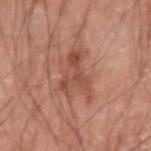The lesion was photographed on a routine skin check and not biopsied; there is no pathology result.
On the right upper arm.
The total-body-photography lesion software estimated a footprint of about 9.5 mm² and an eccentricity of roughly 0.8. The analysis additionally found a border-irregularity rating of about 8/10, internal color variation of about 4 on a 0–10 scale, and radial color variation of about 1. It also reported a classifier nevus-likeness of about 0/100 and a lesion-detection confidence of about 100/100.
A lesion tile, about 15 mm wide, cut from a 3D total-body photograph.
About 5.5 mm across.
Imaged with white-light lighting.
A male patient aged 73–77.